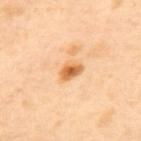Part of a total-body skin-imaging series; this lesion was reviewed on a skin check and was not flagged for biopsy. Automated image analysis of the tile measured a shape eccentricity near 0.75 and two-axis asymmetry of about 0.25. The analysis additionally found a classifier nevus-likeness of about 90/100 and a lesion-detection confidence of about 100/100. The recorded lesion diameter is about 3 mm. The tile uses cross-polarized illumination. From the upper back. A 15 mm crop from a total-body photograph taken for skin-cancer surveillance. A female subject in their 40s.